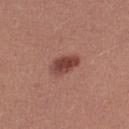Context: Automated tile analysis of the lesion measured a lesion area of about 5.5 mm², a shape eccentricity near 0.8, and a shape-asymmetry score of about 0.25 (0 = symmetric). And it measured a classifier nevus-likeness of about 95/100. A female patient in their mid-20s. About 3.5 mm across. A region of skin cropped from a whole-body photographic capture, roughly 15 mm wide. Imaged with white-light lighting. From the right thigh.